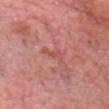Imaged during a routine full-body skin examination; the lesion was not biopsied and no histopathology is available. Automated image analysis of the tile measured radial color variation of about 0. About 3 mm across. The lesion is located on the head or neck. A male patient, aged 78–82. A lesion tile, about 15 mm wide, cut from a 3D total-body photograph. Imaged with white-light lighting.